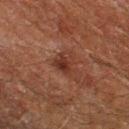Part of a total-body skin-imaging series; this lesion was reviewed on a skin check and was not flagged for biopsy.
Captured under cross-polarized illumination.
Located on the left thigh.
A region of skin cropped from a whole-body photographic capture, roughly 15 mm wide.
A male subject, approximately 60 years of age.
The lesion's longest dimension is about 3 mm.